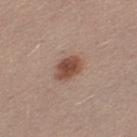The lesion was photographed on a routine skin check and not biopsied; there is no pathology result.
This is a white-light tile.
The lesion's longest dimension is about 3.5 mm.
This image is a 15 mm lesion crop taken from a total-body photograph.
Automated tile analysis of the lesion measured a footprint of about 6.5 mm², an outline eccentricity of about 0.7 (0 = round, 1 = elongated), and a shape-asymmetry score of about 0.15 (0 = symmetric). And it measured a normalized border contrast of about 9.5. The software also gave border irregularity of about 1.5 on a 0–10 scale, internal color variation of about 3.5 on a 0–10 scale, and radial color variation of about 1.
From the left thigh.
A female subject, in their mid- to late 20s.